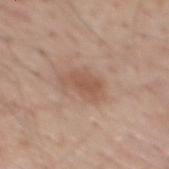Clinical impression:
Imaged during a routine full-body skin examination; the lesion was not biopsied and no histopathology is available.
Acquisition and patient details:
A male subject aged around 65. The lesion is on the mid back. The lesion-visualizer software estimated a lesion color around L≈54 a*≈20 b*≈29 in CIELAB, roughly 9 lightness units darker than nearby skin, and a normalized lesion–skin contrast near 6. A 15 mm close-up extracted from a 3D total-body photography capture. Approximately 4 mm at its widest.